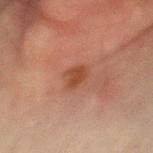Case summary:
– notes — catalogued during a skin exam; not biopsied
– subject — female, about 50 years old
– site — the chest
– image — ~15 mm tile from a whole-body skin photo
– lesion size — ≈2.5 mm
– automated lesion analysis — a border-irregularity index near 2.5/10, internal color variation of about 2.5 on a 0–10 scale, and peripheral color asymmetry of about 1; a nevus-likeness score of about 65/100 and a detector confidence of about 100 out of 100 that the crop contains a lesion
– lighting — cross-polarized